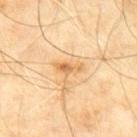The lesion was photographed on a routine skin check and not biopsied; there is no pathology result.
A male subject approximately 60 years of age.
Automated image analysis of the tile measured a footprint of about 4 mm², an eccentricity of roughly 0.9, and two-axis asymmetry of about 0.25. The software also gave border irregularity of about 3 on a 0–10 scale, a within-lesion color-variation index near 3/10, and radial color variation of about 1. The analysis additionally found lesion-presence confidence of about 100/100.
About 3 mm across.
A lesion tile, about 15 mm wide, cut from a 3D total-body photograph.
From the back.